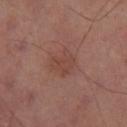<lesion>
<biopsy_status>not biopsied; imaged during a skin examination</biopsy_status>
<lesion_size>
  <long_diameter_mm_approx>3.5</long_diameter_mm_approx>
</lesion_size>
<image>
  <source>total-body photography crop</source>
  <field_of_view_mm>15</field_of_view_mm>
</image>
<automated_metrics>
  <area_mm2_approx>7.5</area_mm2_approx>
  <eccentricity>0.55</eccentricity>
  <border_irregularity_0_10>3.0</border_irregularity_0_10>
  <color_variation_0_10>2.0</color_variation_0_10>
  <peripheral_color_asymmetry>0.5</peripheral_color_asymmetry>
</automated_metrics>
<site>right thigh</site>
</lesion>